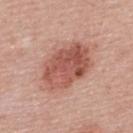Imaged during a routine full-body skin examination; the lesion was not biopsied and no histopathology is available. The lesion is located on the upper back. A female patient, aged around 50. A roughly 15 mm field-of-view crop from a total-body skin photograph. Measured at roughly 6.5 mm in maximum diameter.